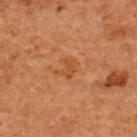| field | value |
|---|---|
| biopsy status | no biopsy performed (imaged during a skin exam) |
| location | the back |
| patient | male, roughly 65 years of age |
| illumination | cross-polarized illumination |
| image | 15 mm crop, total-body photography |
| diameter | about 2.5 mm |